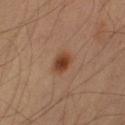Captured during whole-body skin photography for melanoma surveillance; the lesion was not biopsied. Imaged with cross-polarized lighting. Measured at roughly 3 mm in maximum diameter. A 15 mm crop from a total-body photograph taken for skin-cancer surveillance. On the right upper arm. The patient is a male in their mid- to late 40s. The lesion-visualizer software estimated border irregularity of about 1.5 on a 0–10 scale and peripheral color asymmetry of about 1.